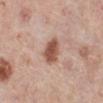This lesion was catalogued during total-body skin photography and was not selected for biopsy. The recorded lesion diameter is about 3.5 mm. The lesion is located on the leg. This is a white-light tile. A female patient about 65 years old. A 15 mm close-up extracted from a 3D total-body photography capture.